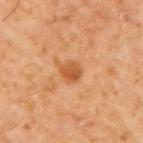| feature | finding |
|---|---|
| notes | catalogued during a skin exam; not biopsied |
| lighting | cross-polarized |
| subject | male, aged 58 to 62 |
| location | the right upper arm |
| diameter | ≈2.5 mm |
| image source | 15 mm crop, total-body photography |